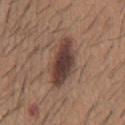The lesion was photographed on a routine skin check and not biopsied; there is no pathology result. A male subject, aged approximately 60. A 15 mm crop from a total-body photograph taken for skin-cancer surveillance. On the mid back. Automated tile analysis of the lesion measured an eccentricity of roughly 0.8 and a shape-asymmetry score of about 0.2 (0 = symmetric). The analysis additionally found a lesion–skin lightness drop of about 13 and a normalized lesion–skin contrast near 11. The software also gave a border-irregularity rating of about 2/10, a color-variation rating of about 6.5/10, and radial color variation of about 1.5. The analysis additionally found a classifier nevus-likeness of about 95/100 and a lesion-detection confidence of about 100/100. Measured at roughly 6 mm in maximum diameter. This is a white-light tile.